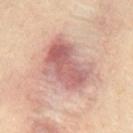<tbp_lesion>
<biopsy_status>not biopsied; imaged during a skin examination</biopsy_status>
<patient>
  <sex>male</sex>
  <age_approx>35</age_approx>
</patient>
<image>
  <source>total-body photography crop</source>
  <field_of_view_mm>15</field_of_view_mm>
</image>
<site>back</site>
</tbp_lesion>